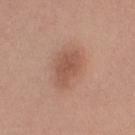Impression: Part of a total-body skin-imaging series; this lesion was reviewed on a skin check and was not flagged for biopsy. Acquisition and patient details: On the right upper arm. A roughly 15 mm field-of-view crop from a total-body skin photograph. A male patient, aged 28 to 32. Captured under white-light illumination. Longest diameter approximately 4 mm.